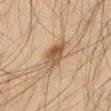Captured during whole-body skin photography for melanoma surveillance; the lesion was not biopsied.
Automated tile analysis of the lesion measured a border-irregularity rating of about 3/10, a color-variation rating of about 6/10, and radial color variation of about 2. The software also gave a classifier nevus-likeness of about 85/100 and lesion-presence confidence of about 100/100.
A region of skin cropped from a whole-body photographic capture, roughly 15 mm wide.
Captured under cross-polarized illumination.
Approximately 5 mm at its widest.
A male subject, about 35 years old.
The lesion is on the right thigh.